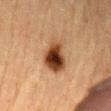This lesion was catalogued during total-body skin photography and was not selected for biopsy.
The lesion is located on the arm.
Captured under cross-polarized illumination.
The patient is a male about 85 years old or older.
Cropped from a whole-body photographic skin survey; the tile spans about 15 mm.
Measured at roughly 5.5 mm in maximum diameter.
An algorithmic analysis of the crop reported a lesion-detection confidence of about 100/100.